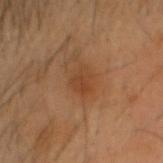Clinical impression: Recorded during total-body skin imaging; not selected for excision or biopsy. Image and clinical context: The total-body-photography lesion software estimated an area of roughly 4.5 mm², an eccentricity of roughly 0.55, and a symmetry-axis asymmetry near 0.25. The software also gave a mean CIELAB color near L≈38 a*≈21 b*≈32 and a lesion–skin lightness drop of about 6. And it measured a nevus-likeness score of about 35/100. This is a cross-polarized tile. Cropped from a whole-body photographic skin survey; the tile spans about 15 mm. Measured at roughly 2.5 mm in maximum diameter. A male subject, roughly 55 years of age. Located on the head or neck.